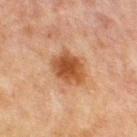Captured during whole-body skin photography for melanoma surveillance; the lesion was not biopsied. From the chest. The lesion's longest dimension is about 4.5 mm. A 15 mm close-up extracted from a 3D total-body photography capture. A male subject in their mid- to late 60s. Imaged with cross-polarized lighting. The lesion-visualizer software estimated a mean CIELAB color near L≈41 a*≈21 b*≈32, a lesion–skin lightness drop of about 11, and a lesion-to-skin contrast of about 10 (normalized; higher = more distinct). It also reported a within-lesion color-variation index near 4/10.